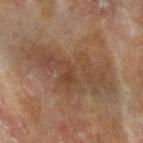Q: What kind of image is this?
A: ~15 mm tile from a whole-body skin photo
Q: Lesion location?
A: the left forearm
Q: Who is the patient?
A: female, aged 73–77
Q: How was the tile lit?
A: cross-polarized
Q: Lesion size?
A: ≈11 mm